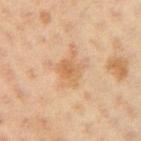This lesion was catalogued during total-body skin photography and was not selected for biopsy. A close-up tile cropped from a whole-body skin photograph, about 15 mm across. Captured under cross-polarized illumination. The lesion is on the right upper arm. A female patient approximately 20 years of age. Measured at roughly 4 mm in maximum diameter.